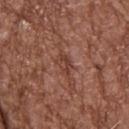- workup: total-body-photography surveillance lesion; no biopsy
- patient: male, in their mid-70s
- image source: total-body-photography crop, ~15 mm field of view
- location: the upper back